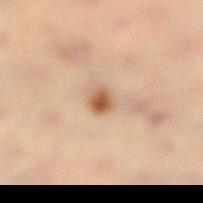Assessment:
The lesion was photographed on a routine skin check and not biopsied; there is no pathology result.
Context:
From the left lower leg. Captured under cross-polarized illumination. Cropped from a whole-body photographic skin survey; the tile spans about 15 mm. Approximately 2 mm at its widest. A female subject in their 30s.